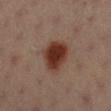  biopsy_status: not biopsied; imaged during a skin examination
  patient:
    sex: female
    age_approx: 20
  site: right lower leg
  image:
    source: total-body photography crop
    field_of_view_mm: 15